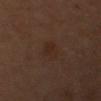Q: Is there a histopathology result?
A: no biopsy performed (imaged during a skin exam)
Q: What are the patient's age and sex?
A: male, approximately 55 years of age
Q: How large is the lesion?
A: ~3 mm (longest diameter)
Q: What is the imaging modality?
A: ~15 mm tile from a whole-body skin photo
Q: Lesion location?
A: the left upper arm
Q: Illumination type?
A: cross-polarized